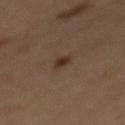Q: Is there a histopathology result?
A: no biopsy performed (imaged during a skin exam)
Q: Lesion location?
A: the mid back
Q: Who is the patient?
A: male, aged 58–62
Q: Illumination type?
A: cross-polarized illumination
Q: What kind of image is this?
A: total-body-photography crop, ~15 mm field of view
Q: What is the lesion's diameter?
A: about 2 mm